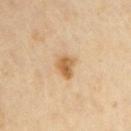biopsy status: imaged on a skin check; not biopsied
anatomic site: the arm
lesion diameter: about 2.5 mm
subject: male, roughly 55 years of age
illumination: cross-polarized
imaging modality: 15 mm crop, total-body photography